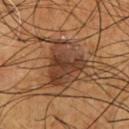Captured during whole-body skin photography for melanoma surveillance; the lesion was not biopsied. Imaged with cross-polarized lighting. From the front of the torso. A male subject in their mid- to late 50s. Longest diameter approximately 5.5 mm. A 15 mm close-up tile from a total-body photography series done for melanoma screening.